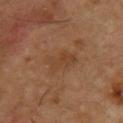{"biopsy_status": "not biopsied; imaged during a skin examination", "automated_metrics": {"cielab_L": 41, "cielab_a": 19, "cielab_b": 31, "vs_skin_darker_L": 5.0}, "site": "chest", "lesion_size": {"long_diameter_mm_approx": 4.0}, "image": {"source": "total-body photography crop", "field_of_view_mm": 15}, "lighting": "cross-polarized", "patient": {"sex": "male", "age_approx": 55}}